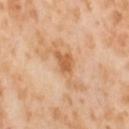Imaged during a routine full-body skin examination; the lesion was not biopsied and no histopathology is available. On the leg. A female subject aged around 55. A roughly 15 mm field-of-view crop from a total-body skin photograph.